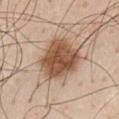| key | value |
|---|---|
| workup | catalogued during a skin exam; not biopsied |
| patient | male, aged 58–62 |
| lesion diameter | ≈5.5 mm |
| image source | ~15 mm crop, total-body skin-cancer survey |
| illumination | white-light illumination |
| automated metrics | a lesion color around L≈52 a*≈20 b*≈32 in CIELAB, a lesion–skin lightness drop of about 16, and a normalized lesion–skin contrast near 11 |
| site | the chest |